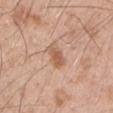This lesion was catalogued during total-body skin photography and was not selected for biopsy. Automated image analysis of the tile measured a lesion-to-skin contrast of about 7 (normalized; higher = more distinct). And it measured border irregularity of about 3.5 on a 0–10 scale, internal color variation of about 2.5 on a 0–10 scale, and peripheral color asymmetry of about 1. Captured under white-light illumination. A male patient in their 50s. The recorded lesion diameter is about 3 mm. On the left upper arm. A roughly 15 mm field-of-view crop from a total-body skin photograph.